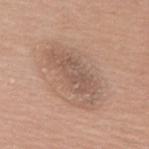Impression: Recorded during total-body skin imaging; not selected for excision or biopsy. Clinical summary: The tile uses white-light illumination. The lesion's longest dimension is about 7 mm. From the upper back. A female patient aged 58–62. A 15 mm crop from a total-body photograph taken for skin-cancer surveillance.